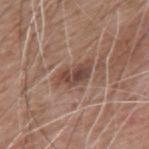Q: Is there a histopathology result?
A: imaged on a skin check; not biopsied
Q: Where on the body is the lesion?
A: the mid back
Q: Illumination type?
A: white-light
Q: Patient demographics?
A: male, aged approximately 60
Q: How was this image acquired?
A: ~15 mm crop, total-body skin-cancer survey